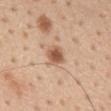The lesion was photographed on a routine skin check and not biopsied; there is no pathology result.
Located on the mid back.
The patient is a male about 55 years old.
A 15 mm close-up extracted from a 3D total-body photography capture.